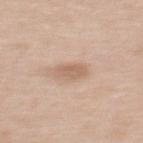Assessment: The lesion was tiled from a total-body skin photograph and was not biopsied. Image and clinical context: Automated tile analysis of the lesion measured an area of roughly 4.5 mm², an eccentricity of roughly 0.75, and a shape-asymmetry score of about 0.15 (0 = symmetric). The analysis additionally found a mean CIELAB color near L≈62 a*≈18 b*≈30, about 9 CIELAB-L* units darker than the surrounding skin, and a normalized lesion–skin contrast near 6. The analysis additionally found a border-irregularity index near 1.5/10 and peripheral color asymmetry of about 1. A 15 mm crop from a total-body photograph taken for skin-cancer surveillance. On the upper back. Measured at roughly 3 mm in maximum diameter. A female patient aged 38–42.